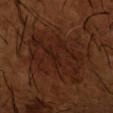Recorded during total-body skin imaging; not selected for excision or biopsy.
The recorded lesion diameter is about 7.5 mm.
An algorithmic analysis of the crop reported an average lesion color of about L≈22 a*≈20 b*≈25 (CIELAB) and a lesion-to-skin contrast of about 6 (normalized; higher = more distinct). The analysis additionally found a border-irregularity index near 5.5/10, a color-variation rating of about 3.5/10, and a peripheral color-asymmetry measure near 1.5.
Cropped from a whole-body photographic skin survey; the tile spans about 15 mm.
Imaged with cross-polarized lighting.
The lesion is on the left forearm.
A male subject, aged approximately 65.